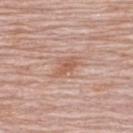Q: Was this lesion biopsied?
A: total-body-photography surveillance lesion; no biopsy
Q: How was this image acquired?
A: 15 mm crop, total-body photography
Q: Where on the body is the lesion?
A: the back
Q: What are the patient's age and sex?
A: female, aged approximately 65
Q: Lesion size?
A: about 3 mm
Q: What lighting was used for the tile?
A: white-light illumination
Q: What did automated image analysis measure?
A: an average lesion color of about L≈57 a*≈22 b*≈30 (CIELAB), roughly 9 lightness units darker than nearby skin, and a normalized border contrast of about 6.5; border irregularity of about 3.5 on a 0–10 scale, internal color variation of about 2 on a 0–10 scale, and radial color variation of about 0.5; a classifier nevus-likeness of about 0/100 and lesion-presence confidence of about 100/100